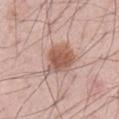notes = total-body-photography surveillance lesion; no biopsy
size = ≈5.5 mm
acquisition = 15 mm crop, total-body photography
TBP lesion metrics = a footprint of about 11 mm² and a symmetry-axis asymmetry near 0.25; lesion-presence confidence of about 100/100
patient = male, aged 53–57
tile lighting = white-light illumination
location = the left thigh